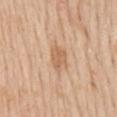Captured during whole-body skin photography for melanoma surveillance; the lesion was not biopsied.
From the mid back.
A 15 mm close-up tile from a total-body photography series done for melanoma screening.
About 3.5 mm across.
A male patient aged around 60.